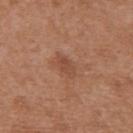This image is a 15 mm lesion crop taken from a total-body photograph. A female patient, aged 38–42. On the upper back.